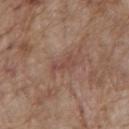Imaged during a routine full-body skin examination; the lesion was not biopsied and no histopathology is available.
A roughly 15 mm field-of-view crop from a total-body skin photograph.
The subject is a male about 70 years old.
The tile uses white-light illumination.
The total-body-photography lesion software estimated a lesion area of about 2.5 mm², a shape eccentricity near 0.95, and a symmetry-axis asymmetry near 0.45.
From the mid back.
Approximately 3 mm at its widest.